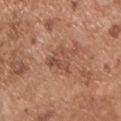biopsy status = no biopsy performed (imaged during a skin exam)
automated lesion analysis = a symmetry-axis asymmetry near 0.45; a mean CIELAB color near L≈50 a*≈21 b*≈30, roughly 8 lightness units darker than nearby skin, and a normalized border contrast of about 6; a border-irregularity rating of about 4.5/10, internal color variation of about 4 on a 0–10 scale, and peripheral color asymmetry of about 1.5
tile lighting = white-light illumination
size = ~3.5 mm (longest diameter)
location = the chest
patient = male, about 55 years old
image source = total-body-photography crop, ~15 mm field of view